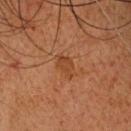{
  "biopsy_status": "not biopsied; imaged during a skin examination",
  "lighting": "cross-polarized",
  "site": "head or neck",
  "patient": {
    "sex": "male",
    "age_approx": 65
  },
  "lesion_size": {
    "long_diameter_mm_approx": 2.5
  },
  "image": {
    "source": "total-body photography crop",
    "field_of_view_mm": 15
  }
}